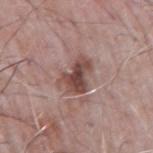<record>
  <patient>
    <sex>male</sex>
    <age_approx>65</age_approx>
  </patient>
  <image>
    <source>total-body photography crop</source>
    <field_of_view_mm>15</field_of_view_mm>
  </image>
  <lesion_size>
    <long_diameter_mm_approx>4.5</long_diameter_mm_approx>
  </lesion_size>
  <lighting>white-light</lighting>
  <site>right upper arm</site>
  <automated_metrics>
    <area_mm2_approx>9.5</area_mm2_approx>
    <eccentricity>0.75</eccentricity>
    <cielab_L>47</cielab_L>
    <cielab_a>20</cielab_a>
    <cielab_b>22</cielab_b>
    <vs_skin_darker_L>12.0</vs_skin_darker_L>
  </automated_metrics>
</record>